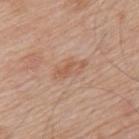The lesion was photographed on a routine skin check and not biopsied; there is no pathology result.